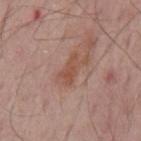Part of a total-body skin-imaging series; this lesion was reviewed on a skin check and was not flagged for biopsy.
The patient is a male aged 63 to 67.
Located on the mid back.
Cropped from a total-body skin-imaging series; the visible field is about 15 mm.